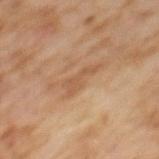Impression: The lesion was tiled from a total-body skin photograph and was not biopsied. Image and clinical context: A female subject, aged 58 to 62. The lesion is located on the upper back. Cropped from a total-body skin-imaging series; the visible field is about 15 mm. The lesion-visualizer software estimated an area of roughly 5 mm². And it measured an average lesion color of about L≈48 a*≈18 b*≈30 (CIELAB), roughly 6 lightness units darker than nearby skin, and a lesion-to-skin contrast of about 5 (normalized; higher = more distinct). The software also gave internal color variation of about 1 on a 0–10 scale and peripheral color asymmetry of about 0.5.